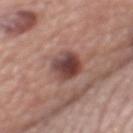The lesion was tiled from a total-body skin photograph and was not biopsied.
A lesion tile, about 15 mm wide, cut from a 3D total-body photograph.
A male subject, aged around 75.
From the mid back.